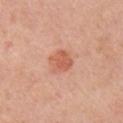Assessment: The lesion was tiled from a total-body skin photograph and was not biopsied. Image and clinical context: Imaged with white-light lighting. Cropped from a whole-body photographic skin survey; the tile spans about 15 mm. Automated image analysis of the tile measured a lesion area of about 6 mm², a shape eccentricity near 0.3, and two-axis asymmetry of about 0.2. The analysis additionally found an automated nevus-likeness rating near 80 out of 100 and a lesion-detection confidence of about 100/100. A female patient about 40 years old. The lesion is located on the arm. Approximately 3 mm at its widest.